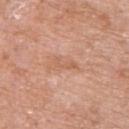<case>
<biopsy_status>not biopsied; imaged during a skin examination</biopsy_status>
<patient>
  <sex>female</sex>
  <age_approx>75</age_approx>
</patient>
<image>
  <source>total-body photography crop</source>
  <field_of_view_mm>15</field_of_view_mm>
</image>
<lighting>white-light</lighting>
<site>back</site>
<lesion_size>
  <long_diameter_mm_approx>3.0</long_diameter_mm_approx>
</lesion_size>
<automated_metrics>
  <area_mm2_approx>3.0</area_mm2_approx>
  <eccentricity>0.95</eccentricity>
  <shape_asymmetry>0.5</shape_asymmetry>
  <vs_skin_darker_L>7.0</vs_skin_darker_L>
  <vs_skin_contrast_norm>5.0</vs_skin_contrast_norm>
</automated_metrics>
</case>